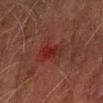– size — ≈4.5 mm
– TBP lesion metrics — border irregularity of about 4 on a 0–10 scale, internal color variation of about 8.5 on a 0–10 scale, and radial color variation of about 3.5; a nevus-likeness score of about 0/100 and a detector confidence of about 100 out of 100 that the crop contains a lesion
– body site — the left forearm
– illumination — cross-polarized
– image — ~15 mm crop, total-body skin-cancer survey
– patient — male, aged around 60